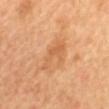Assessment:
Recorded during total-body skin imaging; not selected for excision or biopsy.
Acquisition and patient details:
A female subject, aged around 60. On the mid back. Imaged with cross-polarized lighting. A region of skin cropped from a whole-body photographic capture, roughly 15 mm wide.